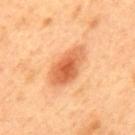This lesion was catalogued during total-body skin photography and was not selected for biopsy. The lesion's longest dimension is about 5.5 mm. A lesion tile, about 15 mm wide, cut from a 3D total-body photograph. On the back. Automated tile analysis of the lesion measured an area of roughly 13 mm² and an eccentricity of roughly 0.85. And it measured an average lesion color of about L≈64 a*≈30 b*≈43 (CIELAB), a lesion–skin lightness drop of about 14, and a normalized lesion–skin contrast near 8. The analysis additionally found a lesion-detection confidence of about 100/100. The tile uses cross-polarized illumination. The patient is a male aged 48 to 52.